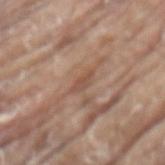  biopsy_status: not biopsied; imaged during a skin examination
  site: back
  lesion_size:
    long_diameter_mm_approx: 2.5
  lighting: white-light
  image:
    source: total-body photography crop
    field_of_view_mm: 15
  patient:
    sex: male
    age_approx: 80